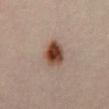The lesion is on the abdomen. Approximately 4 mm at its widest. A male subject, aged 33–37. Captured under cross-polarized illumination. A region of skin cropped from a whole-body photographic capture, roughly 15 mm wide.